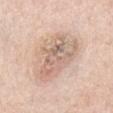No biopsy was performed on this lesion — it was imaged during a full skin examination and was not determined to be concerning.